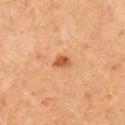Recorded during total-body skin imaging; not selected for excision or biopsy.
Imaged with cross-polarized lighting.
Located on the right upper arm.
A 15 mm close-up tile from a total-body photography series done for melanoma screening.
A female patient in their mid- to late 40s.